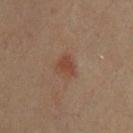Approximately 2.5 mm at its widest.
A male patient aged 33–37.
A 15 mm crop from a total-body photograph taken for skin-cancer surveillance.
The tile uses cross-polarized illumination.
From the upper back.